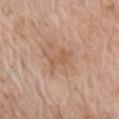  lesion_size:
    long_diameter_mm_approx: 3.0
  site: right upper arm
  automated_metrics:
    area_mm2_approx: 4.5
    eccentricity: 0.8
    shape_asymmetry: 0.3
  image:
    source: total-body photography crop
    field_of_view_mm: 15
  patient:
    sex: female
    age_approx: 75
  lighting: white-light